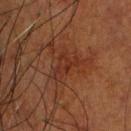notes: total-body-photography surveillance lesion; no biopsy
lesion diameter: ≈4 mm
image: total-body-photography crop, ~15 mm field of view
patient: male, in their mid-80s or older
tile lighting: cross-polarized
site: the head or neck